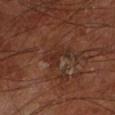Case summary:
- subject · male, aged around 65
- image-analysis metrics · an area of roughly 3 mm², an eccentricity of roughly 0.8, and a shape-asymmetry score of about 0.6 (0 = symmetric); an automated nevus-likeness rating near 0 out of 100 and lesion-presence confidence of about 80/100
- imaging modality · total-body-photography crop, ~15 mm field of view
- location · the left lower leg